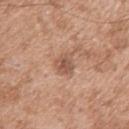biopsy status — imaged on a skin check; not biopsied
TBP lesion metrics — an average lesion color of about L≈55 a*≈21 b*≈29 (CIELAB), roughly 10 lightness units darker than nearby skin, and a lesion-to-skin contrast of about 7 (normalized; higher = more distinct); a border-irregularity rating of about 2/10, internal color variation of about 4.5 on a 0–10 scale, and radial color variation of about 1.5
lesion size — ≈3 mm
lighting — white-light
subject — male, aged approximately 50
site — the right upper arm
image — 15 mm crop, total-body photography